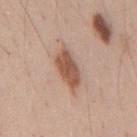Q: Is there a histopathology result?
A: catalogued during a skin exam; not biopsied
Q: How was this image acquired?
A: total-body-photography crop, ~15 mm field of view
Q: What are the patient's age and sex?
A: male, aged around 40
Q: What is the lesion's diameter?
A: ~4.5 mm (longest diameter)
Q: What is the anatomic site?
A: the mid back
Q: What did automated image analysis measure?
A: a classifier nevus-likeness of about 95/100 and a lesion-detection confidence of about 100/100